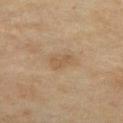Clinical impression:
Recorded during total-body skin imaging; not selected for excision or biopsy.
Clinical summary:
On the mid back. The tile uses cross-polarized illumination. Cropped from a total-body skin-imaging series; the visible field is about 15 mm. A male patient, approximately 60 years of age.